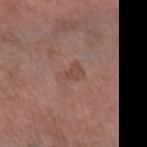Clinical impression: Captured during whole-body skin photography for melanoma surveillance; the lesion was not biopsied.